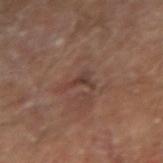Recorded during total-body skin imaging; not selected for excision or biopsy.
The lesion is on the arm.
The patient is a male about 65 years old.
About 3 mm across.
Cropped from a whole-body photographic skin survey; the tile spans about 15 mm.
This is a cross-polarized tile.